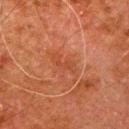Case summary:
- biopsy status: imaged on a skin check; not biopsied
- TBP lesion metrics: a within-lesion color-variation index near 1/10 and a peripheral color-asymmetry measure near 0; a classifier nevus-likeness of about 0/100 and a lesion-detection confidence of about 100/100
- subject: male, approximately 80 years of age
- tile lighting: cross-polarized illumination
- site: the arm
- imaging modality: total-body-photography crop, ~15 mm field of view